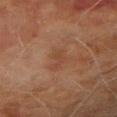illumination: cross-polarized; image source: ~15 mm crop, total-body skin-cancer survey; subject: male, in their 70s; location: the right forearm.